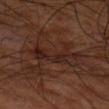<case>
<biopsy_status>not biopsied; imaged during a skin examination</biopsy_status>
<automated_metrics>
  <cielab_L>23</cielab_L>
  <cielab_a>19</cielab_a>
  <cielab_b>22</cielab_b>
  <vs_skin_darker_L>6.0</vs_skin_darker_L>
  <vs_skin_contrast_norm>6.5</vs_skin_contrast_norm>
  <border_irregularity_0_10>9.5</border_irregularity_0_10>
  <color_variation_0_10>3.5</color_variation_0_10>
  <peripheral_color_asymmetry>1.0</peripheral_color_asymmetry>
  <nevus_likeness_0_100>0</nevus_likeness_0_100>
  <lesion_detection_confidence_0_100>95</lesion_detection_confidence_0_100>
</automated_metrics>
<patient>
  <sex>male</sex>
  <age_approx>65</age_approx>
</patient>
<lesion_size>
  <long_diameter_mm_approx>6.0</long_diameter_mm_approx>
</lesion_size>
<site>arm</site>
<lighting>cross-polarized</lighting>
<image>
  <source>total-body photography crop</source>
  <field_of_view_mm>15</field_of_view_mm>
</image>
</case>